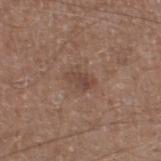Imaged during a routine full-body skin examination; the lesion was not biopsied and no histopathology is available.